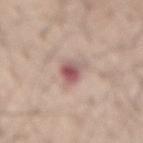The lesion's longest dimension is about 3.5 mm.
The lesion is located on the back.
A male patient, aged around 55.
Imaged with white-light lighting.
A 15 mm close-up tile from a total-body photography series done for melanoma screening.
Automated image analysis of the tile measured an eccentricity of roughly 0.85 and a symmetry-axis asymmetry near 0.2. It also reported a lesion–skin lightness drop of about 13 and a normalized border contrast of about 9.